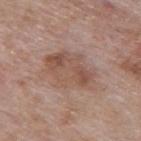{
  "biopsy_status": "not biopsied; imaged during a skin examination",
  "image": {
    "source": "total-body photography crop",
    "field_of_view_mm": 15
  },
  "lighting": "white-light",
  "site": "mid back",
  "patient": {
    "sex": "male",
    "age_approx": 60
  },
  "automated_metrics": {
    "area_mm2_approx": 19.0,
    "eccentricity": 0.75,
    "cielab_L": 52,
    "cielab_a": 18,
    "cielab_b": 26,
    "vs_skin_darker_L": 8.0,
    "color_variation_0_10": 5.0,
    "peripheral_color_asymmetry": 1.5
  }
}